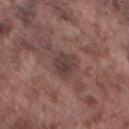Q: Was this lesion biopsied?
A: total-body-photography surveillance lesion; no biopsy
Q: Where on the body is the lesion?
A: the left thigh
Q: Automated lesion metrics?
A: a footprint of about 6.5 mm², a shape eccentricity near 0.7, and a shape-asymmetry score of about 0.25 (0 = symmetric); an average lesion color of about L≈39 a*≈17 b*≈18 (CIELAB), roughly 8 lightness units darker than nearby skin, and a normalized border contrast of about 7; border irregularity of about 2.5 on a 0–10 scale, a within-lesion color-variation index near 3.5/10, and a peripheral color-asymmetry measure near 1.5
Q: How large is the lesion?
A: ≈3.5 mm
Q: Patient demographics?
A: male, aged approximately 75
Q: Illumination type?
A: white-light
Q: How was this image acquired?
A: ~15 mm tile from a whole-body skin photo